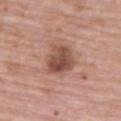This lesion was catalogued during total-body skin photography and was not selected for biopsy.